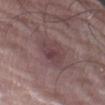| field | value |
|---|---|
| follow-up | imaged on a skin check; not biopsied |
| patient | female, in their mid-60s |
| acquisition | 15 mm crop, total-body photography |
| body site | the abdomen |
| lesion size | ~5 mm (longest diameter) |
| illumination | white-light |
| automated metrics | a lesion area of about 10 mm², an outline eccentricity of about 0.85 (0 = round, 1 = elongated), and two-axis asymmetry of about 0.3; a border-irregularity index near 3/10 and internal color variation of about 3.5 on a 0–10 scale; a classifier nevus-likeness of about 0/100 and a lesion-detection confidence of about 80/100 |